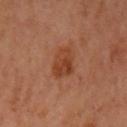Captured during whole-body skin photography for melanoma surveillance; the lesion was not biopsied.